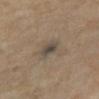Impression: Imaged during a routine full-body skin examination; the lesion was not biopsied and no histopathology is available. Clinical summary: A 15 mm crop from a total-body photograph taken for skin-cancer surveillance. Measured at roughly 2.5 mm in maximum diameter. The tile uses cross-polarized illumination. The lesion is on the right thigh. The subject is a female aged around 50.